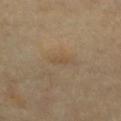Case summary:
- notes — imaged on a skin check; not biopsied
- acquisition — ~15 mm tile from a whole-body skin photo
- patient — approximately 60 years of age
- size — about 2.5 mm
- location — the chest
- lighting — cross-polarized illumination
- TBP lesion metrics — border irregularity of about 2.5 on a 0–10 scale; an automated nevus-likeness rating near 0 out of 100 and a detector confidence of about 95 out of 100 that the crop contains a lesion